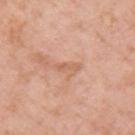The lesion was tiled from a total-body skin photograph and was not biopsied. Cropped from a whole-body photographic skin survey; the tile spans about 15 mm. The patient is a female approximately 70 years of age. Longest diameter approximately 3 mm. This is a white-light tile. The lesion is on the right upper arm.